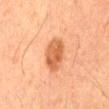  biopsy_status: not biopsied; imaged during a skin examination
  site: mid back
  patient:
    sex: male
    age_approx: 65
  automated_metrics:
    area_mm2_approx: 9.0
    eccentricity: 0.8
    shape_asymmetry: 0.15
    vs_skin_darker_L: 11.0
    vs_skin_contrast_norm: 8.0
  image:
    source: total-body photography crop
    field_of_view_mm: 15